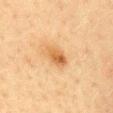Notes:
– notes · no biopsy performed (imaged during a skin exam)
– image · total-body-photography crop, ~15 mm field of view
– TBP lesion metrics · an area of roughly 5 mm², an eccentricity of roughly 0.8, and a symmetry-axis asymmetry near 0.15; an average lesion color of about L≈51 a*≈19 b*≈37 (CIELAB), roughly 10 lightness units darker than nearby skin, and a normalized border contrast of about 7.5; a border-irregularity rating of about 1.5/10 and peripheral color asymmetry of about 2; an automated nevus-likeness rating near 80 out of 100 and lesion-presence confidence of about 100/100
– location · the chest
– illumination · cross-polarized
– subject · female, aged 38 to 42